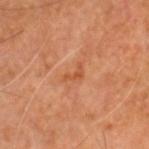{
  "lesion_size": {
    "long_diameter_mm_approx": 2.5
  },
  "image": {
    "source": "total-body photography crop",
    "field_of_view_mm": 15
  },
  "patient": {
    "sex": "male",
    "age_approx": 60
  },
  "site": "head or neck"
}